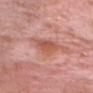biopsy status — total-body-photography surveillance lesion; no biopsy | acquisition — total-body-photography crop, ~15 mm field of view | TBP lesion metrics — a lesion area of about 8.5 mm² and a shape eccentricity near 0.85; a border-irregularity index near 4/10 and a within-lesion color-variation index near 4.5/10 | tile lighting — white-light | body site — the head or neck | patient — male, about 80 years old.